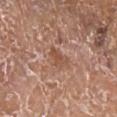| key | value |
|---|---|
| body site | the right lower leg |
| acquisition | ~15 mm crop, total-body skin-cancer survey |
| subject | female, in their mid- to late 70s |